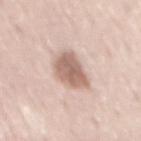Impression:
No biopsy was performed on this lesion — it was imaged during a full skin examination and was not determined to be concerning.
Image and clinical context:
This is a white-light tile. Cropped from a whole-body photographic skin survey; the tile spans about 15 mm. The patient is a male about 50 years old. The lesion is on the mid back. An algorithmic analysis of the crop reported an average lesion color of about L≈63 a*≈18 b*≈25 (CIELAB) and about 15 CIELAB-L* units darker than the surrounding skin. Approximately 4.5 mm at its widest.